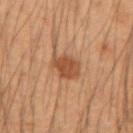The lesion was photographed on a routine skin check and not biopsied; there is no pathology result. From the left forearm. A male subject in their mid- to late 30s. Imaged with cross-polarized lighting. Cropped from a total-body skin-imaging series; the visible field is about 15 mm. The lesion-visualizer software estimated a shape eccentricity near 0.55. The software also gave a border-irregularity rating of about 2.5/10, a color-variation rating of about 2.5/10, and radial color variation of about 1. The recorded lesion diameter is about 3.5 mm.